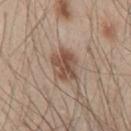biopsy_status: not biopsied; imaged during a skin examination
site: chest
patient:
  sex: male
  age_approx: 45
lesion_size:
  long_diameter_mm_approx: 3.5
image:
  source: total-body photography crop
  field_of_view_mm: 15